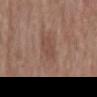workup: total-body-photography surveillance lesion; no biopsy | site: the mid back | subject: male, roughly 70 years of age | acquisition: total-body-photography crop, ~15 mm field of view.